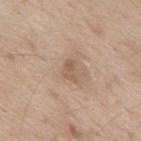{"biopsy_status": "not biopsied; imaged during a skin examination", "patient": {"sex": "male", "age_approx": 65}, "site": "mid back", "lesion_size": {"long_diameter_mm_approx": 3.0}, "image": {"source": "total-body photography crop", "field_of_view_mm": 15}}